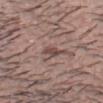Impression: Recorded during total-body skin imaging; not selected for excision or biopsy. Clinical summary: Imaged with white-light lighting. The lesion's longest dimension is about 2.5 mm. A male subject aged 28 to 32. The lesion is located on the head or neck. Cropped from a whole-body photographic skin survey; the tile spans about 15 mm. Automated image analysis of the tile measured an eccentricity of roughly 0.8 and two-axis asymmetry of about 0.3.